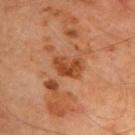Q: Lesion location?
A: the upper back
Q: What did automated image analysis measure?
A: a border-irregularity index near 3/10, a color-variation rating of about 3/10, and radial color variation of about 1
Q: What lighting was used for the tile?
A: cross-polarized
Q: How was this image acquired?
A: total-body-photography crop, ~15 mm field of view
Q: How large is the lesion?
A: ≈3.5 mm
Q: Patient demographics?
A: male, about 65 years old